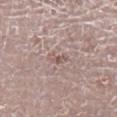Clinical impression: Recorded during total-body skin imaging; not selected for excision or biopsy. Image and clinical context: The lesion is on the right leg. Approximately 2.5 mm at its widest. The subject is a male aged 68 to 72. This is a white-light tile. A 15 mm close-up tile from a total-body photography series done for melanoma screening.